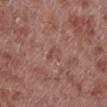A close-up tile cropped from a whole-body skin photograph, about 15 mm across.
Automated image analysis of the tile measured a mean CIELAB color near L≈47 a*≈23 b*≈25, roughly 6 lightness units darker than nearby skin, and a lesion-to-skin contrast of about 4.5 (normalized; higher = more distinct). The software also gave lesion-presence confidence of about 95/100.
A male patient, aged approximately 55.
Captured under white-light illumination.
On the left lower leg.
About 2.5 mm across.